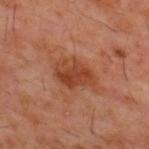notes: catalogued during a skin exam; not biopsied | acquisition: 15 mm crop, total-body photography | anatomic site: the upper back | subject: male, aged approximately 60.